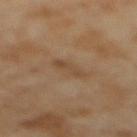Impression: This lesion was catalogued during total-body skin photography and was not selected for biopsy. Context: On the mid back. This is a cross-polarized tile. The patient is a female aged 53–57. Cropped from a total-body skin-imaging series; the visible field is about 15 mm. Automated tile analysis of the lesion measured a footprint of about 4 mm², a shape eccentricity near 0.9, and two-axis asymmetry of about 0.3. It also reported a lesion color around L≈47 a*≈16 b*≈32 in CIELAB, roughly 6 lightness units darker than nearby skin, and a normalized border contrast of about 5.